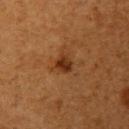{
  "biopsy_status": "not biopsied; imaged during a skin examination",
  "site": "left upper arm",
  "image": {
    "source": "total-body photography crop",
    "field_of_view_mm": 15
  },
  "patient": {
    "sex": "male",
    "age_approx": 45
  },
  "lesion_size": {
    "long_diameter_mm_approx": 2.5
  }
}